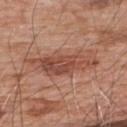Imaged during a routine full-body skin examination; the lesion was not biopsied and no histopathology is available.
The subject is a male aged around 80.
A region of skin cropped from a whole-body photographic capture, roughly 15 mm wide.
Located on the upper back.
The lesion's longest dimension is about 8.5 mm.
The lesion-visualizer software estimated an area of roughly 19 mm² and two-axis asymmetry of about 0.35. And it measured a mean CIELAB color near L≈49 a*≈24 b*≈29 and a normalized border contrast of about 7. The analysis additionally found a nevus-likeness score of about 80/100 and a detector confidence of about 95 out of 100 that the crop contains a lesion.
Captured under white-light illumination.